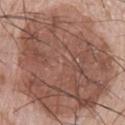Q: Is there a histopathology result?
A: catalogued during a skin exam; not biopsied
Q: What is the lesion's diameter?
A: about 16.5 mm
Q: Where on the body is the lesion?
A: the left upper arm
Q: What are the patient's age and sex?
A: male, about 55 years old
Q: How was this image acquired?
A: ~15 mm tile from a whole-body skin photo